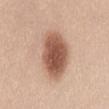Captured during whole-body skin photography for melanoma surveillance; the lesion was not biopsied.
A female patient, about 20 years old.
Longest diameter approximately 7 mm.
This is a white-light tile.
Automated tile analysis of the lesion measured an area of roughly 20 mm² and a shape eccentricity near 0.85. And it measured a normalized lesion–skin contrast near 10.5. The analysis additionally found a border-irregularity rating of about 2/10, internal color variation of about 5 on a 0–10 scale, and a peripheral color-asymmetry measure near 1.5.
Cropped from a whole-body photographic skin survey; the tile spans about 15 mm.
Located on the abdomen.